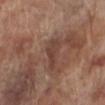Q: Was a biopsy performed?
A: imaged on a skin check; not biopsied
Q: What kind of image is this?
A: ~15 mm tile from a whole-body skin photo
Q: What is the anatomic site?
A: the left lower leg
Q: What lighting was used for the tile?
A: white-light illumination
Q: Automated lesion metrics?
A: an average lesion color of about L≈41 a*≈20 b*≈24 (CIELAB), a lesion–skin lightness drop of about 7, and a normalized lesion–skin contrast near 6; a border-irregularity index near 5/10 and a color-variation rating of about 1.5/10; lesion-presence confidence of about 65/100
Q: What are the patient's age and sex?
A: male, aged around 70